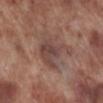Imaged during a routine full-body skin examination; the lesion was not biopsied and no histopathology is available.
The patient is a male approximately 70 years of age.
From the right lower leg.
Approximately 5 mm at its widest.
The total-body-photography lesion software estimated a lesion area of about 13 mm² and two-axis asymmetry of about 0.4. It also reported a normalized border contrast of about 6.5. The software also gave a border-irregularity index near 5/10, a within-lesion color-variation index near 4/10, and a peripheral color-asymmetry measure near 1.
This is a white-light tile.
A 15 mm close-up tile from a total-body photography series done for melanoma screening.